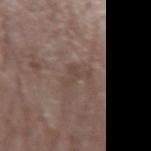Captured under white-light illumination. A region of skin cropped from a whole-body photographic capture, roughly 15 mm wide. From the arm. Approximately 3.5 mm at its widest. The subject is a female about 70 years old. Automated image analysis of the tile measured an area of roughly 3.5 mm². It also reported an average lesion color of about L≈44 a*≈15 b*≈22 (CIELAB) and a normalized border contrast of about 5. It also reported a border-irregularity index near 9/10, a within-lesion color-variation index near 0/10, and peripheral color asymmetry of about 0. It also reported a nevus-likeness score of about 0/100 and a lesion-detection confidence of about 95/100.